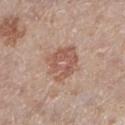Clinical impression:
Captured during whole-body skin photography for melanoma surveillance; the lesion was not biopsied.
Image and clinical context:
On the leg. An algorithmic analysis of the crop reported a footprint of about 13 mm² and a shape-asymmetry score of about 0.25 (0 = symmetric). The analysis additionally found a classifier nevus-likeness of about 20/100. This is a white-light tile. A 15 mm close-up extracted from a 3D total-body photography capture. A female patient aged around 60. Measured at roughly 4.5 mm in maximum diameter.